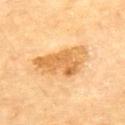workup: total-body-photography surveillance lesion; no biopsy
acquisition: total-body-photography crop, ~15 mm field of view
size: ≈7 mm
site: the upper back
subject: male, aged 83 or older
illumination: cross-polarized illumination